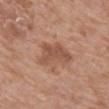Longest diameter approximately 4.5 mm.
A 15 mm crop from a total-body photograph taken for skin-cancer surveillance.
Imaged with white-light lighting.
The subject is a female aged around 75.
The lesion-visualizer software estimated a lesion–skin lightness drop of about 9 and a normalized lesion–skin contrast near 6.5.
On the mid back.